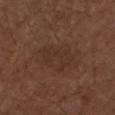Impression: The lesion was photographed on a routine skin check and not biopsied; there is no pathology result. Image and clinical context: This is a white-light tile. An algorithmic analysis of the crop reported a lesion area of about 16 mm², a shape eccentricity near 0.75, and two-axis asymmetry of about 0.2. The analysis additionally found an average lesion color of about L≈32 a*≈18 b*≈24 (CIELAB). It also reported a border-irregularity rating of about 2.5/10, internal color variation of about 1.5 on a 0–10 scale, and radial color variation of about 0.5. The software also gave a classifier nevus-likeness of about 0/100 and a detector confidence of about 100 out of 100 that the crop contains a lesion. A close-up tile cropped from a whole-body skin photograph, about 15 mm across. On the left upper arm. The subject is a male in their mid-70s.